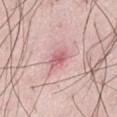This lesion was catalogued during total-body skin photography and was not selected for biopsy. A close-up tile cropped from a whole-body skin photograph, about 15 mm across. The lesion's longest dimension is about 2.5 mm. An algorithmic analysis of the crop reported a lesion area of about 4.5 mm², an eccentricity of roughly 0.6, and a shape-asymmetry score of about 0.2 (0 = symmetric). It also reported an average lesion color of about L≈62 a*≈28 b*≈20 (CIELAB). The software also gave a border-irregularity index near 1.5/10, a within-lesion color-variation index near 3.5/10, and a peripheral color-asymmetry measure near 1. It also reported a nevus-likeness score of about 5/100 and lesion-presence confidence of about 100/100. The lesion is located on the left thigh. This is a white-light tile. A male subject in their 50s.